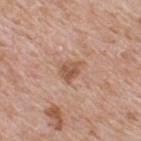size: ~3 mm (longest diameter)
patient: male, roughly 65 years of age
body site: the upper back
image: ~15 mm tile from a whole-body skin photo
TBP lesion metrics: a footprint of about 5 mm², an eccentricity of roughly 0.6, and a symmetry-axis asymmetry near 0.3; an average lesion color of about L≈55 a*≈21 b*≈31 (CIELAB), a lesion–skin lightness drop of about 10, and a normalized lesion–skin contrast near 7; a border-irregularity rating of about 3/10 and a color-variation rating of about 3/10